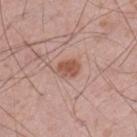Q: Is there a histopathology result?
A: catalogued during a skin exam; not biopsied
Q: How large is the lesion?
A: ≈2.5 mm
Q: Patient demographics?
A: male, approximately 55 years of age
Q: Automated lesion metrics?
A: a lesion area of about 4 mm², an outline eccentricity of about 0.7 (0 = round, 1 = elongated), and a symmetry-axis asymmetry near 0.2
Q: What is the imaging modality?
A: 15 mm crop, total-body photography
Q: What is the anatomic site?
A: the left thigh
Q: Illumination type?
A: white-light